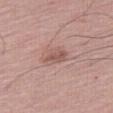follow-up: no biopsy performed (imaged during a skin exam); diameter: ~2.5 mm (longest diameter); image source: 15 mm crop, total-body photography; subject: male, in their mid-60s; location: the leg.